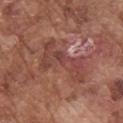Captured during whole-body skin photography for melanoma surveillance; the lesion was not biopsied.
This image is a 15 mm lesion crop taken from a total-body photograph.
This is a white-light tile.
The lesion-visualizer software estimated a lesion area of about 13 mm², a shape eccentricity near 0.9, and a shape-asymmetry score of about 0.6 (0 = symmetric). It also reported an average lesion color of about L≈44 a*≈24 b*≈25 (CIELAB). The analysis additionally found a border-irregularity index near 9/10 and a peripheral color-asymmetry measure near 1.5.
Approximately 7 mm at its widest.
On the arm.
The subject is a male in their mid- to late 70s.